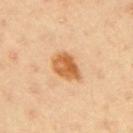follow-up = imaged on a skin check; not biopsied
image source = ~15 mm crop, total-body skin-cancer survey
location = the right upper arm
lighting = cross-polarized
subject = male, in their 40s
lesion diameter = ≈3.5 mm
TBP lesion metrics = a lesion color around L≈62 a*≈25 b*≈44 in CIELAB and a lesion–skin lightness drop of about 14; a border-irregularity index near 2/10, a within-lesion color-variation index near 6/10, and a peripheral color-asymmetry measure near 2; a nevus-likeness score of about 100/100 and lesion-presence confidence of about 100/100